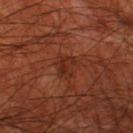{
  "biopsy_status": "not biopsied; imaged during a skin examination",
  "image": {
    "source": "total-body photography crop",
    "field_of_view_mm": 15
  },
  "site": "left thigh",
  "patient": {
    "sex": "male",
    "age_approx": 70
  },
  "lesion_size": {
    "long_diameter_mm_approx": 2.5
  },
  "automated_metrics": {
    "area_mm2_approx": 5.0,
    "eccentricity": 0.55,
    "shape_asymmetry": 0.35,
    "cielab_L": 28,
    "cielab_a": 25,
    "cielab_b": 29,
    "vs_skin_darker_L": 7.0,
    "color_variation_0_10": 3.5,
    "peripheral_color_asymmetry": 1.0,
    "nevus_likeness_0_100": 0,
    "lesion_detection_confidence_0_100": 95
  },
  "lighting": "cross-polarized"
}